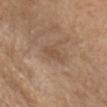Context:
Approximately 2.5 mm at its widest. Located on the head or neck. An algorithmic analysis of the crop reported a lesion–skin lightness drop of about 6 and a lesion-to-skin contrast of about 5 (normalized; higher = more distinct). The software also gave a classifier nevus-likeness of about 0/100 and a lesion-detection confidence of about 100/100. A roughly 15 mm field-of-view crop from a total-body skin photograph. Imaged with white-light lighting. A male subject roughly 70 years of age.